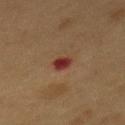Notes:
• automated lesion analysis — a shape eccentricity near 0.7 and a shape-asymmetry score of about 0.25 (0 = symmetric); a lesion color around L≈31 a*≈25 b*≈24 in CIELAB, about 11 CIELAB-L* units darker than the surrounding skin, and a normalized border contrast of about 10.5
• imaging modality — total-body-photography crop, ~15 mm field of view
• tile lighting — cross-polarized
• size — ~2.5 mm (longest diameter)
• location — the back
• patient — male, approximately 55 years of age